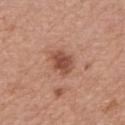Impression: Recorded during total-body skin imaging; not selected for excision or biopsy. Image and clinical context: Cropped from a total-body skin-imaging series; the visible field is about 15 mm. The tile uses white-light illumination. The lesion is located on the right forearm. The total-body-photography lesion software estimated a within-lesion color-variation index near 3/10 and radial color variation of about 1. The analysis additionally found an automated nevus-likeness rating near 85 out of 100 and lesion-presence confidence of about 100/100. The subject is a female aged approximately 60.